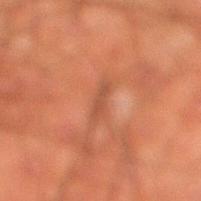notes: imaged on a skin check; not biopsied
imaging modality: total-body-photography crop, ~15 mm field of view
lesion size: ≈3 mm
tile lighting: cross-polarized illumination
location: the right lower leg
patient: male, aged around 50
TBP lesion metrics: an average lesion color of about L≈40 a*≈23 b*≈29 (CIELAB), a lesion–skin lightness drop of about 6, and a lesion-to-skin contrast of about 5.5 (normalized; higher = more distinct); a border-irregularity index near 4.5/10, internal color variation of about 0 on a 0–10 scale, and radial color variation of about 0; a classifier nevus-likeness of about 0/100